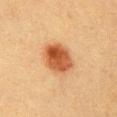Assessment: No biopsy was performed on this lesion — it was imaged during a full skin examination and was not determined to be concerning. Context: Captured under cross-polarized illumination. The lesion is on the chest. A region of skin cropped from a whole-body photographic capture, roughly 15 mm wide. Automated image analysis of the tile measured an area of roughly 12 mm² and a shape-asymmetry score of about 0.1 (0 = symmetric). It also reported a lesion-to-skin contrast of about 10 (normalized; higher = more distinct). And it measured a within-lesion color-variation index near 5.5/10 and radial color variation of about 2. The subject is a female aged approximately 40. The recorded lesion diameter is about 4 mm.